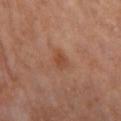Clinical impression: Imaged during a routine full-body skin examination; the lesion was not biopsied and no histopathology is available. Clinical summary: Measured at roughly 2.5 mm in maximum diameter. Imaged with cross-polarized lighting. The patient is a female aged approximately 50. The lesion is located on the left thigh. A close-up tile cropped from a whole-body skin photograph, about 15 mm across.